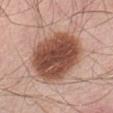Clinical impression:
Captured during whole-body skin photography for melanoma surveillance; the lesion was not biopsied.
Acquisition and patient details:
Measured at roughly 8 mm in maximum diameter. A lesion tile, about 15 mm wide, cut from a 3D total-body photograph. A male patient, aged 53–57. On the abdomen. The lesion-visualizer software estimated a mean CIELAB color near L≈49 a*≈22 b*≈28, roughly 18 lightness units darker than nearby skin, and a normalized lesion–skin contrast near 12. And it measured border irregularity of about 1.5 on a 0–10 scale, a color-variation rating of about 5.5/10, and a peripheral color-asymmetry measure near 2. The tile uses white-light illumination.